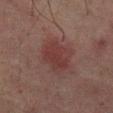Q: Was a biopsy performed?
A: no biopsy performed (imaged during a skin exam)
Q: What is the anatomic site?
A: the mid back
Q: Who is the patient?
A: male, aged approximately 65
Q: What kind of image is this?
A: ~15 mm tile from a whole-body skin photo
Q: How was the tile lit?
A: cross-polarized
Q: Automated lesion metrics?
A: a lesion area of about 12 mm², an outline eccentricity of about 0.75 (0 = round, 1 = elongated), and a symmetry-axis asymmetry near 0.2; a mean CIELAB color near L≈28 a*≈18 b*≈17, a lesion–skin lightness drop of about 6, and a lesion-to-skin contrast of about 6.5 (normalized; higher = more distinct); a border-irregularity index near 2.5/10 and a within-lesion color-variation index near 2.5/10; a nevus-likeness score of about 55/100